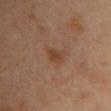Clinical impression:
Part of a total-body skin-imaging series; this lesion was reviewed on a skin check and was not flagged for biopsy.
Image and clinical context:
About 3 mm across. The patient is a female aged around 40. This is a cross-polarized tile. A roughly 15 mm field-of-view crop from a total-body skin photograph. The lesion is located on the chest. Automated image analysis of the tile measured an area of roughly 4 mm², an eccentricity of roughly 0.8, and two-axis asymmetry of about 0.25.